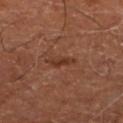No biopsy was performed on this lesion — it was imaged during a full skin examination and was not determined to be concerning.
From the left lower leg.
Automated image analysis of the tile measured a footprint of about 3.5 mm², a shape eccentricity near 0.9, and a symmetry-axis asymmetry near 0.35. And it measured a mean CIELAB color near L≈35 a*≈23 b*≈29 and a normalized lesion–skin contrast near 7. It also reported border irregularity of about 4 on a 0–10 scale and radial color variation of about 0.
The patient is aged 63–67.
Cropped from a whole-body photographic skin survey; the tile spans about 15 mm.